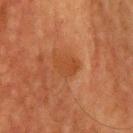{
  "biopsy_status": "not biopsied; imaged during a skin examination",
  "site": "head or neck",
  "automated_metrics": {
    "area_mm2_approx": 6.0,
    "eccentricity": 0.55,
    "border_irregularity_0_10": 2.0,
    "nevus_likeness_0_100": 0
  },
  "lighting": "cross-polarized",
  "patient": {
    "sex": "male",
    "age_approx": 60
  },
  "lesion_size": {
    "long_diameter_mm_approx": 3.0
  },
  "image": {
    "source": "total-body photography crop",
    "field_of_view_mm": 15
  }
}